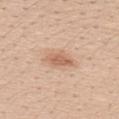biopsy status = imaged on a skin check; not biopsied | subject = female, in their mid- to late 20s | size = ~3.5 mm (longest diameter) | acquisition = total-body-photography crop, ~15 mm field of view | body site = the front of the torso | TBP lesion metrics = a lesion area of about 5 mm² and two-axis asymmetry of about 0.25; a mean CIELAB color near L≈62 a*≈21 b*≈32, about 10 CIELAB-L* units darker than the surrounding skin, and a normalized border contrast of about 7; an automated nevus-likeness rating near 75 out of 100.